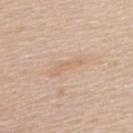{
  "image": {
    "source": "total-body photography crop",
    "field_of_view_mm": 15
  },
  "patient": {
    "sex": "female",
    "age_approx": 60
  },
  "site": "upper back",
  "lesion_size": {
    "long_diameter_mm_approx": 4.5
  },
  "automated_metrics": {
    "area_mm2_approx": 5.0,
    "eccentricity": 0.95,
    "shape_asymmetry": 0.45
  },
  "lighting": "white-light"
}